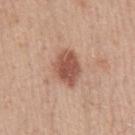Assessment: No biopsy was performed on this lesion — it was imaged during a full skin examination and was not determined to be concerning. Context: The tile uses white-light illumination. A close-up tile cropped from a whole-body skin photograph, about 15 mm across. A male subject aged around 30. Measured at roughly 4.5 mm in maximum diameter. From the left upper arm.